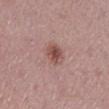biopsy_status: not biopsied; imaged during a skin examination
patient:
  sex: male
  age_approx: 65
site: mid back
image:
  source: total-body photography crop
  field_of_view_mm: 15